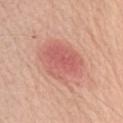Q: Is there a histopathology result?
A: imaged on a skin check; not biopsied
Q: What is the anatomic site?
A: the right upper arm
Q: Who is the patient?
A: male, aged around 70
Q: How was the tile lit?
A: white-light illumination
Q: What did automated image analysis measure?
A: a lesion area of about 21 mm², an outline eccentricity of about 0.6 (0 = round, 1 = elongated), and a shape-asymmetry score of about 0.15 (0 = symmetric); a classifier nevus-likeness of about 50/100
Q: What is the imaging modality?
A: 15 mm crop, total-body photography